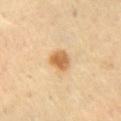Assessment: The lesion was tiled from a total-body skin photograph and was not biopsied. Context: Located on the lower back. The tile uses cross-polarized illumination. A close-up tile cropped from a whole-body skin photograph, about 15 mm across. A female subject about 65 years old.